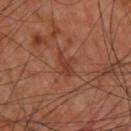biopsy_status: not biopsied; imaged during a skin examination
automated_metrics:
  area_mm2_approx: 4.0
  shape_asymmetry: 0.4
  cielab_L: 38
  cielab_a: 24
  cielab_b: 29
  vs_skin_darker_L: 7.0
  vs_skin_contrast_norm: 6.0
patient:
  sex: male
  age_approx: 60
image:
  source: total-body photography crop
  field_of_view_mm: 15
lighting: cross-polarized
site: upper back